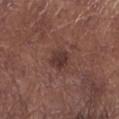biopsy status = imaged on a skin check; not biopsied | body site = the leg | subject = male, in their mid- to late 50s | image source = total-body-photography crop, ~15 mm field of view | tile lighting = white-light | lesion size = ~2.5 mm (longest diameter).